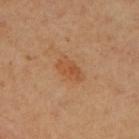Impression:
Recorded during total-body skin imaging; not selected for excision or biopsy.
Image and clinical context:
A female patient in their 60s. Automated tile analysis of the lesion measured an eccentricity of roughly 0.85 and a shape-asymmetry score of about 0.25 (0 = symmetric). The software also gave a border-irregularity rating of about 2.5/10 and a within-lesion color-variation index near 3/10. Imaged with cross-polarized lighting. A 15 mm close-up extracted from a 3D total-body photography capture. Approximately 3.5 mm at its widest. On the right upper arm.